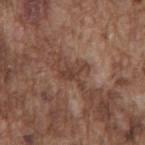notes: total-body-photography surveillance lesion; no biopsy
anatomic site: the mid back
subject: male, aged around 75
illumination: white-light illumination
image source: ~15 mm crop, total-body skin-cancer survey